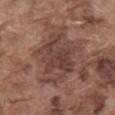Clinical impression: The lesion was photographed on a routine skin check and not biopsied; there is no pathology result. Background: The subject is a male in their mid-70s. Measured at roughly 7.5 mm in maximum diameter. Located on the mid back. A 15 mm close-up tile from a total-body photography series done for melanoma screening. The tile uses white-light illumination.